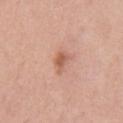On the chest. The lesion-visualizer software estimated a shape eccentricity near 0.75 and a symmetry-axis asymmetry near 0.35. The tile uses white-light illumination. The lesion's longest dimension is about 2.5 mm. A male patient roughly 55 years of age. A lesion tile, about 15 mm wide, cut from a 3D total-body photograph.